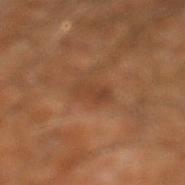notes: catalogued during a skin exam; not biopsied
patient: male, aged approximately 60
lighting: cross-polarized illumination
body site: the right leg
image-analysis metrics: a lesion area of about 5 mm², a shape eccentricity near 0.9, and two-axis asymmetry of about 0.15; a detector confidence of about 100 out of 100 that the crop contains a lesion
imaging modality: ~15 mm crop, total-body skin-cancer survey
lesion diameter: about 4 mm